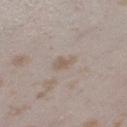Part of a total-body skin-imaging series; this lesion was reviewed on a skin check and was not flagged for biopsy. Located on the left thigh. A female patient aged 23 to 27. An algorithmic analysis of the crop reported a lesion area of about 4 mm² and a shape-asymmetry score of about 0.25 (0 = symmetric). The analysis additionally found a mean CIELAB color near L≈58 a*≈12 b*≈23 and a normalized border contrast of about 5. The analysis additionally found a border-irregularity rating of about 2.5/10 and peripheral color asymmetry of about 0.5. Imaged with white-light lighting. Cropped from a whole-body photographic skin survey; the tile spans about 15 mm.